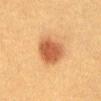notes: imaged on a skin check; not biopsied | lighting: cross-polarized illumination | subject: female, aged around 40 | imaging modality: 15 mm crop, total-body photography | lesion size: ≈4 mm | TBP lesion metrics: a lesion color around L≈47 a*≈23 b*≈34 in CIELAB and a lesion-to-skin contrast of about 9 (normalized; higher = more distinct) | site: the abdomen.